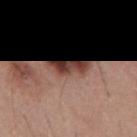Acquisition and patient details:
Approximately 5.5 mm at its widest. Imaged with white-light lighting. A male subject, roughly 55 years of age. Located on the mid back. Cropped from a whole-body photographic skin survey; the tile spans about 15 mm.
Conclusion:
The lesion was biopsied, and histopathology showed a dysplastic (Clark) nevus, classified as a benign skin lesion.